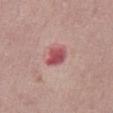Findings:
- notes · imaged on a skin check; not biopsied
- image · ~15 mm crop, total-body skin-cancer survey
- subject · male, aged approximately 75
- site · the abdomen
- tile lighting · white-light